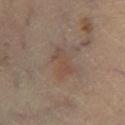{"biopsy_status": "not biopsied; imaged during a skin examination", "site": "leg", "patient": {"age_approx": 60}, "lighting": "cross-polarized", "automated_metrics": {"area_mm2_approx": 6.5, "border_irregularity_0_10": 4.5, "color_variation_0_10": 3.5, "nevus_likeness_0_100": 10, "lesion_detection_confidence_0_100": 100}, "lesion_size": {"long_diameter_mm_approx": 4.0}, "image": {"source": "total-body photography crop", "field_of_view_mm": 15}}